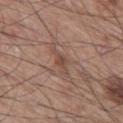Recorded during total-body skin imaging; not selected for excision or biopsy.
Captured under white-light illumination.
A lesion tile, about 15 mm wide, cut from a 3D total-body photograph.
A male subject, roughly 60 years of age.
The lesion is on the leg.